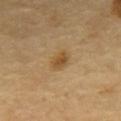biopsy_status: not biopsied; imaged during a skin examination
site: back
patient:
  sex: male
  age_approx: 65
image:
  source: total-body photography crop
  field_of_view_mm: 15